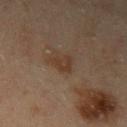Imaged during a routine full-body skin examination; the lesion was not biopsied and no histopathology is available. A roughly 15 mm field-of-view crop from a total-body skin photograph. The patient is a male in their mid- to late 40s. Located on the left upper arm.